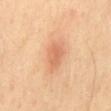Part of a total-body skin-imaging series; this lesion was reviewed on a skin check and was not flagged for biopsy. The patient is a male about 50 years old. This is a cross-polarized tile. Located on the mid back. A roughly 15 mm field-of-view crop from a total-body skin photograph. An algorithmic analysis of the crop reported an automated nevus-likeness rating near 45 out of 100.